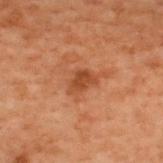Q: Is there a histopathology result?
A: no biopsy performed (imaged during a skin exam)
Q: Who is the patient?
A: male, roughly 70 years of age
Q: How was the tile lit?
A: cross-polarized illumination
Q: Automated lesion metrics?
A: a border-irregularity rating of about 3.5/10 and peripheral color asymmetry of about 0.5; a classifier nevus-likeness of about 15/100 and lesion-presence confidence of about 100/100
Q: What kind of image is this?
A: 15 mm crop, total-body photography
Q: Lesion location?
A: the back